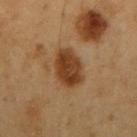| feature | finding |
|---|---|
| biopsy status | total-body-photography surveillance lesion; no biopsy |
| subject | male, about 60 years old |
| lighting | cross-polarized |
| site | the right upper arm |
| diameter | ≈4.5 mm |
| image | 15 mm crop, total-body photography |
| automated lesion analysis | a mean CIELAB color near L≈39 a*≈21 b*≈34, about 13 CIELAB-L* units darker than the surrounding skin, and a normalized lesion–skin contrast near 10.5; a border-irregularity rating of about 2/10 |